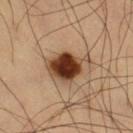  biopsy_status: not biopsied; imaged during a skin examination
  patient:
    sex: male
    age_approx: 60
  site: right thigh
  lighting: cross-polarized
  image:
    source: total-body photography crop
    field_of_view_mm: 15
  lesion_size:
    long_diameter_mm_approx: 5.0
  automated_metrics:
    area_mm2_approx: 13.0
    eccentricity: 0.7
    shape_asymmetry: 0.25
    nevus_likeness_0_100: 100
    lesion_detection_confidence_0_100: 100